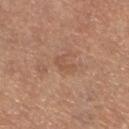Captured during whole-body skin photography for melanoma surveillance; the lesion was not biopsied.